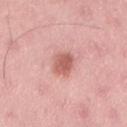Clinical impression:
Part of a total-body skin-imaging series; this lesion was reviewed on a skin check and was not flagged for biopsy.
Clinical summary:
Located on the back. Cropped from a whole-body photographic skin survey; the tile spans about 15 mm. Automated tile analysis of the lesion measured a lesion area of about 6 mm² and two-axis asymmetry of about 0.25. The software also gave an average lesion color of about L≈60 a*≈27 b*≈26 (CIELAB) and roughly 12 lightness units darker than nearby skin. It also reported a classifier nevus-likeness of about 80/100 and lesion-presence confidence of about 100/100. This is a white-light tile. The subject is a female about 50 years old.